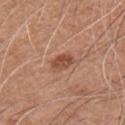follow-up: total-body-photography surveillance lesion; no biopsy | imaging modality: total-body-photography crop, ~15 mm field of view | lesion size: ≈3 mm | patient: male, roughly 60 years of age | automated metrics: an eccentricity of roughly 0.75; a mean CIELAB color near L≈50 a*≈24 b*≈31, a lesion–skin lightness drop of about 10, and a lesion-to-skin contrast of about 7.5 (normalized; higher = more distinct); a border-irregularity index near 1.5/10, a within-lesion color-variation index near 3/10, and peripheral color asymmetry of about 1 | site: the chest | illumination: white-light.